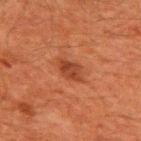Q: How was this image acquired?
A: ~15 mm tile from a whole-body skin photo
Q: Where on the body is the lesion?
A: the upper back
Q: Patient demographics?
A: male, roughly 60 years of age
Q: What is the lesion's diameter?
A: ≈3 mm
Q: What lighting was used for the tile?
A: cross-polarized illumination